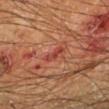This lesion was catalogued during total-body skin photography and was not selected for biopsy. Imaged with cross-polarized lighting. A roughly 15 mm field-of-view crop from a total-body skin photograph. An algorithmic analysis of the crop reported a footprint of about 2.5 mm², an outline eccentricity of about 0.9 (0 = round, 1 = elongated), and two-axis asymmetry of about 0.4. And it measured a mean CIELAB color near L≈36 a*≈27 b*≈27 and about 7 CIELAB-L* units darker than the surrounding skin. The lesion is on the left lower leg. The patient is a male aged approximately 65.